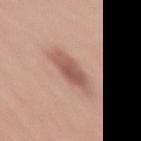Background: Measured at roughly 4.5 mm in maximum diameter. The lesion is located on the leg. This is a white-light tile. A male subject, aged around 50. Cropped from a whole-body photographic skin survey; the tile spans about 15 mm.